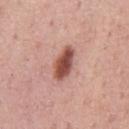Imaged during a routine full-body skin examination; the lesion was not biopsied and no histopathology is available. Automated tile analysis of the lesion measured a footprint of about 8.5 mm², a shape eccentricity near 0.85, and a symmetry-axis asymmetry near 0.15. And it measured a border-irregularity index near 1.5/10. The lesion's longest dimension is about 4.5 mm. A 15 mm close-up tile from a total-body photography series done for melanoma screening. A male subject aged approximately 75. The lesion is located on the front of the torso.